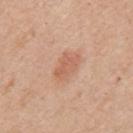<case>
  <lighting>white-light</lighting>
  <site>mid back</site>
  <image>
    <source>total-body photography crop</source>
    <field_of_view_mm>15</field_of_view_mm>
  </image>
  <patient>
    <sex>male</sex>
    <age_approx>55</age_approx>
  </patient>
</case>